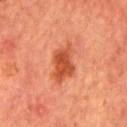Findings:
* biopsy status · imaged on a skin check; not biopsied
* tile lighting · cross-polarized
* patient · male, approximately 65 years of age
* image source · 15 mm crop, total-body photography
* location · the front of the torso
* automated metrics · a footprint of about 10 mm², an outline eccentricity of about 0.85 (0 = round, 1 = elongated), and a symmetry-axis asymmetry near 0.35; a lesion color around L≈47 a*≈32 b*≈37 in CIELAB and about 12 CIELAB-L* units darker than the surrounding skin; a classifier nevus-likeness of about 95/100
* diameter · ≈5 mm